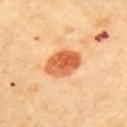Captured during whole-body skin photography for melanoma surveillance; the lesion was not biopsied. The tile uses cross-polarized illumination. The lesion is on the back. The patient is a female about 60 years old. Measured at roughly 4.5 mm in maximum diameter. Cropped from a whole-body photographic skin survey; the tile spans about 15 mm.